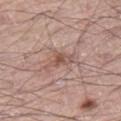Case summary:
* follow-up: catalogued during a skin exam; not biopsied
* patient: male, in their 70s
* size: ~3.5 mm (longest diameter)
* image source: 15 mm crop, total-body photography
* lighting: white-light
* anatomic site: the leg